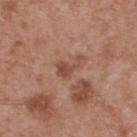The lesion was photographed on a routine skin check and not biopsied; there is no pathology result.
The tile uses white-light illumination.
The subject is a male in their mid-50s.
Located on the upper back.
A roughly 15 mm field-of-view crop from a total-body skin photograph.
Longest diameter approximately 3 mm.